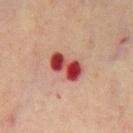The lesion was photographed on a routine skin check and not biopsied; there is no pathology result.
A close-up tile cropped from a whole-body skin photograph, about 15 mm across.
The lesion is located on the mid back.
The subject is a male approximately 70 years of age.